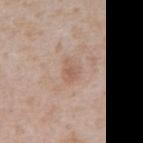No biopsy was performed on this lesion — it was imaged during a full skin examination and was not determined to be concerning.
Longest diameter approximately 2.5 mm.
The lesion is located on the abdomen.
Captured under white-light illumination.
Cropped from a whole-body photographic skin survey; the tile spans about 15 mm.
A male patient aged 63–67.